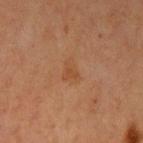A female subject, roughly 40 years of age. Cropped from a whole-body photographic skin survey; the tile spans about 15 mm. The lesion is on the arm.